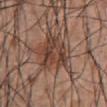- workup: total-body-photography surveillance lesion; no biopsy
- lighting: white-light
- image: ~15 mm crop, total-body skin-cancer survey
- size: ~4 mm (longest diameter)
- subject: male, aged 53–57
- location: the abdomen